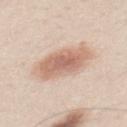Recorded during total-body skin imaging; not selected for excision or biopsy.